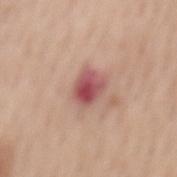Part of a total-body skin-imaging series; this lesion was reviewed on a skin check and was not flagged for biopsy. The tile uses white-light illumination. A lesion tile, about 15 mm wide, cut from a 3D total-body photograph. A female subject aged around 65. Located on the mid back.